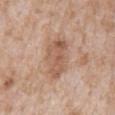Q: Was a biopsy performed?
A: imaged on a skin check; not biopsied
Q: How was this image acquired?
A: ~15 mm crop, total-body skin-cancer survey
Q: What is the anatomic site?
A: the chest
Q: How large is the lesion?
A: about 5.5 mm
Q: What are the patient's age and sex?
A: male, aged approximately 65
Q: How was the tile lit?
A: white-light